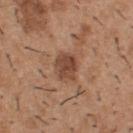| field | value |
|---|---|
| biopsy status | no biopsy performed (imaged during a skin exam) |
| image-analysis metrics | a classifier nevus-likeness of about 50/100 and lesion-presence confidence of about 100/100 |
| diameter | about 3 mm |
| subject | male, aged 38–42 |
| illumination | white-light |
| anatomic site | the upper back |
| acquisition | 15 mm crop, total-body photography |